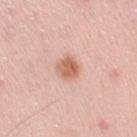workup: catalogued during a skin exam; not biopsied | body site: the right upper arm | acquisition: 15 mm crop, total-body photography | diameter: ≈2.5 mm | lighting: white-light | subject: female, in their 40s.